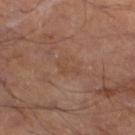Captured during whole-body skin photography for melanoma surveillance; the lesion was not biopsied.
The lesion is located on the leg.
Longest diameter approximately 3 mm.
A male patient aged approximately 55.
A 15 mm close-up extracted from a 3D total-body photography capture.
Captured under cross-polarized illumination.